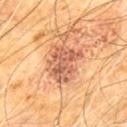Notes:
– notes — no biopsy performed (imaged during a skin exam)
– imaging modality — ~15 mm tile from a whole-body skin photo
– subject — male, aged 58–62
– site — the chest